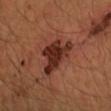Impression: Recorded during total-body skin imaging; not selected for excision or biopsy. Context: Longest diameter approximately 6 mm. Captured under cross-polarized illumination. A male subject in their 40s. Located on the right forearm. This image is a 15 mm lesion crop taken from a total-body photograph.